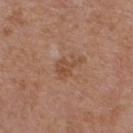• workup — total-body-photography surveillance lesion; no biopsy
• TBP lesion metrics — a lesion color around L≈48 a*≈21 b*≈31 in CIELAB; a color-variation rating of about 2.5/10 and a peripheral color-asymmetry measure near 1; a nevus-likeness score of about 0/100 and lesion-presence confidence of about 100/100
• site — the chest
• lesion size — ≈3.5 mm
• patient — male, aged 53–57
• image — 15 mm crop, total-body photography
• tile lighting — white-light